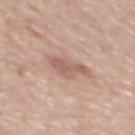{
  "biopsy_status": "not biopsied; imaged during a skin examination",
  "image": {
    "source": "total-body photography crop",
    "field_of_view_mm": 15
  },
  "site": "upper back",
  "lighting": "white-light",
  "lesion_size": {
    "long_diameter_mm_approx": 4.0
  },
  "patient": {
    "sex": "male",
    "age_approx": 60
  }
}